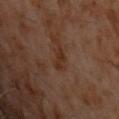Recorded during total-body skin imaging; not selected for excision or biopsy.
A male subject, about 60 years old.
On the chest.
A close-up tile cropped from a whole-body skin photograph, about 15 mm across.
Captured under cross-polarized illumination.
Automated tile analysis of the lesion measured a mean CIELAB color near L≈28 a*≈17 b*≈26 and about 6 CIELAB-L* units darker than the surrounding skin. The software also gave border irregularity of about 3 on a 0–10 scale, a color-variation rating of about 2/10, and radial color variation of about 0.5.
Approximately 3 mm at its widest.